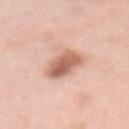Q: Was this lesion biopsied?
A: catalogued during a skin exam; not biopsied
Q: Illumination type?
A: white-light
Q: Automated lesion metrics?
A: a footprint of about 10 mm² and a shape eccentricity near 0.9; an automated nevus-likeness rating near 80 out of 100 and a lesion-detection confidence of about 100/100
Q: Patient demographics?
A: female, aged approximately 50
Q: Lesion size?
A: ~5.5 mm (longest diameter)
Q: What is the anatomic site?
A: the mid back
Q: How was this image acquired?
A: 15 mm crop, total-body photography